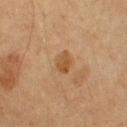workup: total-body-photography surveillance lesion; no biopsy | TBP lesion metrics: border irregularity of about 2 on a 0–10 scale, internal color variation of about 2.5 on a 0–10 scale, and a peripheral color-asymmetry measure near 1 | site: the left upper arm | imaging modality: total-body-photography crop, ~15 mm field of view | size: about 3 mm | patient: male, aged 53 to 57.